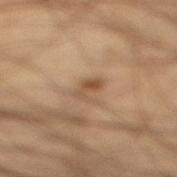notes: total-body-photography surveillance lesion; no biopsy
image: ~15 mm crop, total-body skin-cancer survey
patient: male, aged 43–47
size: ≈3 mm
illumination: cross-polarized illumination
site: the right lower leg